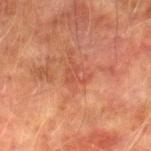| feature | finding |
|---|---|
| biopsy status | no biopsy performed (imaged during a skin exam) |
| diameter | ≈2.5 mm |
| anatomic site | the right lower leg |
| imaging modality | 15 mm crop, total-body photography |
| image-analysis metrics | an area of roughly 3.5 mm², a shape eccentricity near 0.65, and a shape-asymmetry score of about 0.7 (0 = symmetric); a normalized border contrast of about 4.5; border irregularity of about 7.5 on a 0–10 scale and a peripheral color-asymmetry measure near 0.5; a classifier nevus-likeness of about 0/100 and lesion-presence confidence of about 100/100 |
| illumination | cross-polarized illumination |
| patient | male, about 75 years old |